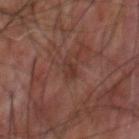<lesion>
<biopsy_status>not biopsied; imaged during a skin examination</biopsy_status>
<image>
  <source>total-body photography crop</source>
  <field_of_view_mm>15</field_of_view_mm>
</image>
<site>upper back</site>
<patient>
  <sex>male</sex>
  <age_approx>60</age_approx>
</patient>
<lighting>cross-polarized</lighting>
</lesion>